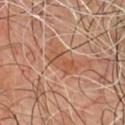Impression:
No biopsy was performed on this lesion — it was imaged during a full skin examination and was not determined to be concerning.
Image and clinical context:
This is a cross-polarized tile. A male subject, about 70 years old. On the chest. A lesion tile, about 15 mm wide, cut from a 3D total-body photograph. Longest diameter approximately 4 mm.